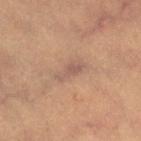Located on the right thigh.
A close-up tile cropped from a whole-body skin photograph, about 15 mm across.
The patient is a female aged around 30.
The recorded lesion diameter is about 4 mm.